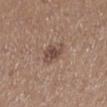Recorded during total-body skin imaging; not selected for excision or biopsy.
The patient is a female aged approximately 40.
A region of skin cropped from a whole-body photographic capture, roughly 15 mm wide.
Measured at roughly 3 mm in maximum diameter.
Captured under white-light illumination.
On the left lower leg.